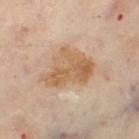follow-up=no biopsy performed (imaged during a skin exam)